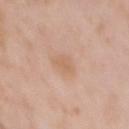Recorded during total-body skin imaging; not selected for excision or biopsy.
On the chest.
A 15 mm close-up tile from a total-body photography series done for melanoma screening.
A female patient, approximately 50 years of age.
Automated image analysis of the tile measured a normalized lesion–skin contrast near 5. The software also gave a border-irregularity rating of about 2.5/10, internal color variation of about 2 on a 0–10 scale, and peripheral color asymmetry of about 0.5.
The recorded lesion diameter is about 3.5 mm.